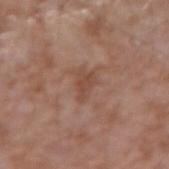Q: Was this lesion biopsied?
A: no biopsy performed (imaged during a skin exam)
Q: Patient demographics?
A: male, aged 73 to 77
Q: What is the anatomic site?
A: the right forearm
Q: How was this image acquired?
A: 15 mm crop, total-body photography